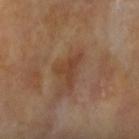No biopsy was performed on this lesion — it was imaged during a full skin examination and was not determined to be concerning.
The lesion is on the right forearm.
An algorithmic analysis of the crop reported border irregularity of about 7 on a 0–10 scale, internal color variation of about 2.5 on a 0–10 scale, and peripheral color asymmetry of about 1. The analysis additionally found a nevus-likeness score of about 5/100 and lesion-presence confidence of about 100/100.
A close-up tile cropped from a whole-body skin photograph, about 15 mm across.
The recorded lesion diameter is about 4 mm.
A female subject, in their mid-70s.
The tile uses cross-polarized illumination.